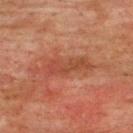biopsy status = imaged on a skin check; not biopsied | patient = male, in their mid-70s | anatomic site = the upper back | illumination = cross-polarized illumination | acquisition = ~15 mm crop, total-body skin-cancer survey.